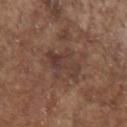Image and clinical context:
The lesion's longest dimension is about 5 mm. A region of skin cropped from a whole-body photographic capture, roughly 15 mm wide. Automated tile analysis of the lesion measured a mean CIELAB color near L≈38 a*≈17 b*≈23. The patient is a male in their 80s. The lesion is located on the head or neck.